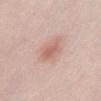notes — no biopsy performed (imaged during a skin exam) | tile lighting — white-light illumination | anatomic site — the abdomen | patient — male, aged 48–52 | size — about 4 mm | automated lesion analysis — an area of roughly 8 mm², a shape eccentricity near 0.75, and a symmetry-axis asymmetry near 0.2; a border-irregularity rating of about 2/10 and a peripheral color-asymmetry measure near 1; a classifier nevus-likeness of about 95/100 and a lesion-detection confidence of about 100/100 | acquisition — ~15 mm tile from a whole-body skin photo.